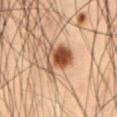Recorded during total-body skin imaging; not selected for excision or biopsy.